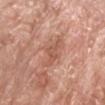Recorded during total-body skin imaging; not selected for excision or biopsy.
Located on the head or neck.
A male patient aged 63 to 67.
Cropped from a whole-body photographic skin survey; the tile spans about 15 mm.